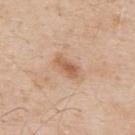biopsy status = total-body-photography surveillance lesion; no biopsy
site = the upper back
tile lighting = white-light illumination
diameter = ≈3 mm
image source = ~15 mm crop, total-body skin-cancer survey
patient = male, aged 58 to 62